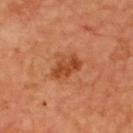Q: Was this lesion biopsied?
A: imaged on a skin check; not biopsied
Q: What lighting was used for the tile?
A: cross-polarized illumination
Q: What is the lesion's diameter?
A: ~4 mm (longest diameter)
Q: What is the imaging modality?
A: ~15 mm tile from a whole-body skin photo
Q: Patient demographics?
A: male, approximately 65 years of age
Q: What did automated image analysis measure?
A: a footprint of about 7.5 mm² and an eccentricity of roughly 0.8; an average lesion color of about L≈48 a*≈29 b*≈40 (CIELAB), roughly 10 lightness units darker than nearby skin, and a normalized border contrast of about 7.5
Q: Where on the body is the lesion?
A: the upper back